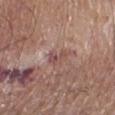Context: Longest diameter approximately 2.5 mm. The patient is a male aged approximately 65. Captured under white-light illumination. This image is a 15 mm lesion crop taken from a total-body photograph. The total-body-photography lesion software estimated a lesion area of about 3 mm², an outline eccentricity of about 0.85 (0 = round, 1 = elongated), and two-axis asymmetry of about 0.5. The analysis additionally found about 8 CIELAB-L* units darker than the surrounding skin and a normalized border contrast of about 6.5. It also reported a classifier nevus-likeness of about 0/100 and a lesion-detection confidence of about 55/100. From the leg.